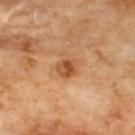Impression:
Recorded during total-body skin imaging; not selected for excision or biopsy.
Background:
A 15 mm close-up extracted from a 3D total-body photography capture. The lesion-visualizer software estimated a border-irregularity rating of about 1.5/10, a color-variation rating of about 4.5/10, and radial color variation of about 1.5. Imaged with cross-polarized lighting. The lesion is located on the upper back. Measured at roughly 2.5 mm in maximum diameter.